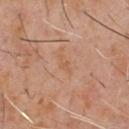| feature | finding |
|---|---|
| follow-up | catalogued during a skin exam; not biopsied |
| subject | male, aged around 60 |
| imaging modality | 15 mm crop, total-body photography |
| anatomic site | the chest |
| tile lighting | cross-polarized |
| automated metrics | a shape eccentricity near 0.9 and a symmetry-axis asymmetry near 0.25; a lesion color around L≈52 a*≈20 b*≈31 in CIELAB, roughly 4 lightness units darker than nearby skin, and a lesion-to-skin contrast of about 4.5 (normalized; higher = more distinct) |